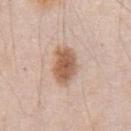Notes:
- notes: total-body-photography surveillance lesion; no biopsy
- lesion diameter: about 5 mm
- image: ~15 mm tile from a whole-body skin photo
- tile lighting: white-light
- patient: male, in their mid-40s
- location: the front of the torso
- image-analysis metrics: an outline eccentricity of about 0.8 (0 = round, 1 = elongated) and a shape-asymmetry score of about 0.2 (0 = symmetric); about 13 CIELAB-L* units darker than the surrounding skin; border irregularity of about 2 on a 0–10 scale and internal color variation of about 4 on a 0–10 scale